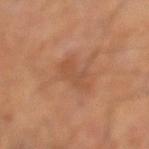| field | value |
|---|---|
| notes | total-body-photography surveillance lesion; no biopsy |
| tile lighting | cross-polarized |
| anatomic site | the left lower leg |
| lesion size | ≈4.5 mm |
| imaging modality | total-body-photography crop, ~15 mm field of view |
| image-analysis metrics | an eccentricity of roughly 0.95 and a shape-asymmetry score of about 0.35 (0 = symmetric); a lesion color around L≈48 a*≈22 b*≈33 in CIELAB and roughly 7 lightness units darker than nearby skin |
| patient | male, in their mid-60s |